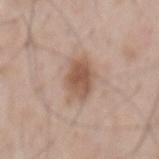biopsy_status: not biopsied; imaged during a skin examination
site: mid back
patient:
  sex: male
  age_approx: 55
image:
  source: total-body photography crop
  field_of_view_mm: 15
lighting: white-light